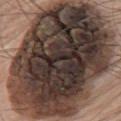Assessment: Imaged during a routine full-body skin examination; the lesion was not biopsied and no histopathology is available. Acquisition and patient details: A male patient, aged approximately 75. Cropped from a whole-body photographic skin survey; the tile spans about 15 mm. The tile uses white-light illumination. The recorded lesion diameter is about 15.5 mm. An algorithmic analysis of the crop reported roughly 23 lightness units darker than nearby skin. The software also gave a border-irregularity rating of about 1.5/10, a color-variation rating of about 10/10, and radial color variation of about 4. From the chest.